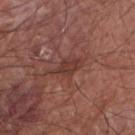Clinical impression:
This lesion was catalogued during total-body skin photography and was not selected for biopsy.
Image and clinical context:
A 15 mm crop from a total-body photograph taken for skin-cancer surveillance. The lesion's longest dimension is about 4.5 mm. The patient is a male in their mid-60s. Automated tile analysis of the lesion measured a border-irregularity index near 4/10. The lesion is located on the right forearm.